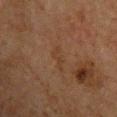<record>
  <biopsy_status>not biopsied; imaged during a skin examination</biopsy_status>
  <lighting>cross-polarized</lighting>
  <site>chest</site>
  <lesion_size>
    <long_diameter_mm_approx>3.5</long_diameter_mm_approx>
  </lesion_size>
  <patient>
    <sex>male</sex>
    <age_approx>50</age_approx>
  </patient>
  <image>
    <source>total-body photography crop</source>
    <field_of_view_mm>15</field_of_view_mm>
  </image>
</record>